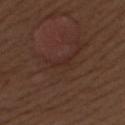Cropped from a whole-body photographic skin survey; the tile spans about 15 mm. A female patient aged approximately 50. The lesion is located on the upper back. Measured at roughly 3 mm in maximum diameter. Imaged with white-light lighting.